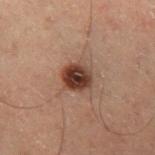{
  "site": "leg",
  "image": {
    "source": "total-body photography crop",
    "field_of_view_mm": 15
  },
  "automated_metrics": {
    "nevus_likeness_0_100": 95,
    "lesion_detection_confidence_0_100": 100
  },
  "patient": {
    "sex": "male",
    "age_approx": 60
  },
  "lighting": "cross-polarized",
  "lesion_size": {
    "long_diameter_mm_approx": 3.5
  }
}